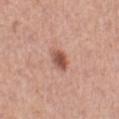Clinical impression:
The lesion was photographed on a routine skin check and not biopsied; there is no pathology result.
Clinical summary:
The lesion-visualizer software estimated a footprint of about 4.5 mm², an outline eccentricity of about 0.75 (0 = round, 1 = elongated), and two-axis asymmetry of about 0.2. The analysis additionally found a lesion–skin lightness drop of about 14 and a normalized border contrast of about 9. And it measured a within-lesion color-variation index near 4/10 and radial color variation of about 1. It also reported lesion-presence confidence of about 100/100. From the left lower leg. Longest diameter approximately 3 mm. A lesion tile, about 15 mm wide, cut from a 3D total-body photograph. A female patient, in their mid-60s. This is a white-light tile.